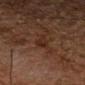Q: Was a biopsy performed?
A: catalogued during a skin exam; not biopsied
Q: Lesion location?
A: the left thigh
Q: What kind of image is this?
A: ~15 mm tile from a whole-body skin photo
Q: Who is the patient?
A: male, aged around 80
Q: Illumination type?
A: cross-polarized illumination
Q: What is the lesion's diameter?
A: ~3.5 mm (longest diameter)